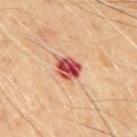Imaged during a routine full-body skin examination; the lesion was not biopsied and no histopathology is available.
Automated tile analysis of the lesion measured an area of roughly 6.5 mm² and a shape-asymmetry score of about 0.2 (0 = symmetric). The software also gave internal color variation of about 10 on a 0–10 scale and a peripheral color-asymmetry measure near 4.
Captured under cross-polarized illumination.
The lesion is on the mid back.
A 15 mm crop from a total-body photograph taken for skin-cancer surveillance.
Measured at roughly 3 mm in maximum diameter.
A male patient, aged 68–72.